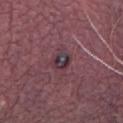biopsy_status: not biopsied; imaged during a skin examination
patient:
  sex: male
  age_approx: 65
lesion_size:
  long_diameter_mm_approx: 2.5
image:
  source: total-body photography crop
  field_of_view_mm: 15
site: abdomen
lighting: white-light
automated_metrics:
  cielab_L: 33
  cielab_a: 17
  cielab_b: 8
  vs_skin_darker_L: 8.0
  vs_skin_contrast_norm: 9.0
  border_irregularity_0_10: 2.0
  color_variation_0_10: 7.5
  lesion_detection_confidence_0_100: 95